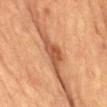Captured during whole-body skin photography for melanoma surveillance; the lesion was not biopsied. The tile uses cross-polarized illumination. Cropped from a whole-body photographic skin survey; the tile spans about 15 mm. The lesion-visualizer software estimated an average lesion color of about L≈46 a*≈22 b*≈33 (CIELAB) and a lesion–skin lightness drop of about 12. The software also gave a border-irregularity rating of about 5.5/10, a color-variation rating of about 3/10, and peripheral color asymmetry of about 1. On the abdomen. A male patient approximately 85 years of age or older.